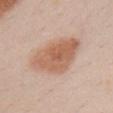Captured during whole-body skin photography for melanoma surveillance; the lesion was not biopsied. This is a white-light tile. From the front of the torso. Cropped from a total-body skin-imaging series; the visible field is about 15 mm. The patient is a female in their mid-20s. An algorithmic analysis of the crop reported an outline eccentricity of about 0.8 (0 = round, 1 = elongated) and two-axis asymmetry of about 0.2.